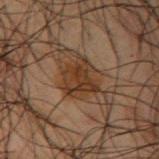The lesion was photographed on a routine skin check and not biopsied; there is no pathology result.
A roughly 15 mm field-of-view crop from a total-body skin photograph.
The lesion-visualizer software estimated an eccentricity of roughly 0.55 and a shape-asymmetry score of about 0.3 (0 = symmetric). It also reported a mean CIELAB color near L≈25 a*≈14 b*≈24, roughly 8 lightness units darker than nearby skin, and a normalized border contrast of about 9. The software also gave an automated nevus-likeness rating near 15 out of 100 and a lesion-detection confidence of about 100/100.
Imaged with cross-polarized lighting.
From the right upper arm.
A male patient about 50 years old.